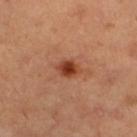<lesion>
<biopsy_status>not biopsied; imaged during a skin examination</biopsy_status>
<image>
  <source>total-body photography crop</source>
  <field_of_view_mm>15</field_of_view_mm>
</image>
<lesion_size>
  <long_diameter_mm_approx>3.0</long_diameter_mm_approx>
</lesion_size>
<patient>
  <sex>female</sex>
  <age_approx>30</age_approx>
</patient>
<site>left lower leg</site>
<lighting>cross-polarized</lighting>
</lesion>